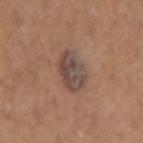Notes:
– tile lighting — white-light
– subject — male, aged 48–52
– anatomic site — the left forearm
– image — ~15 mm tile from a whole-body skin photo